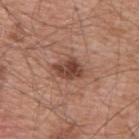| field | value |
|---|---|
| notes | total-body-photography surveillance lesion; no biopsy |